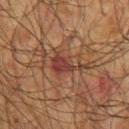notes: catalogued during a skin exam; not biopsied
patient: male, approximately 75 years of age
size: about 4.5 mm
imaging modality: ~15 mm tile from a whole-body skin photo
body site: the arm